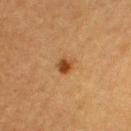Part of a total-body skin-imaging series; this lesion was reviewed on a skin check and was not flagged for biopsy.
Imaged with cross-polarized lighting.
A region of skin cropped from a whole-body photographic capture, roughly 15 mm wide.
The subject is a female aged approximately 55.
Located on the arm.